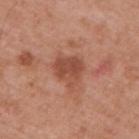Findings:
• biopsy status: total-body-photography surveillance lesion; no biopsy
• lighting: white-light
• image source: 15 mm crop, total-body photography
• location: the upper back
• subject: male, in their mid-50s